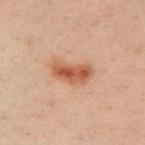  lesion_size:
    long_diameter_mm_approx: 4.0
  automated_metrics:
    cielab_L: 44
    cielab_a: 21
    cielab_b: 28
    vs_skin_darker_L: 10.0
    vs_skin_contrast_norm: 8.5
    border_irregularity_0_10: 3.0
    color_variation_0_10: 5.0
    peripheral_color_asymmetry: 1.5
    lesion_detection_confidence_0_100: 100
  lighting: cross-polarized
  patient:
    sex: male
    age_approx: 40
  site: right upper arm
  image:
    source: total-body photography crop
    field_of_view_mm: 15